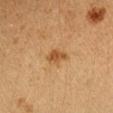The lesion is on the arm. A lesion tile, about 15 mm wide, cut from a 3D total-body photograph. The lesion-visualizer software estimated a lesion area of about 3.5 mm², an eccentricity of roughly 0.85, and a symmetry-axis asymmetry near 0.4. The software also gave a lesion color around L≈45 a*≈20 b*≈36 in CIELAB, about 10 CIELAB-L* units darker than the surrounding skin, and a normalized border contrast of about 8. A female patient, aged 18–22. Measured at roughly 2.5 mm in maximum diameter.